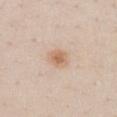Impression:
The lesion was photographed on a routine skin check and not biopsied; there is no pathology result.
Background:
This is a white-light tile. A female subject aged 23–27. A region of skin cropped from a whole-body photographic capture, roughly 15 mm wide. The lesion is on the chest. Automated image analysis of the tile measured a lesion color around L≈65 a*≈18 b*≈32 in CIELAB, a lesion–skin lightness drop of about 9, and a normalized lesion–skin contrast near 7. The analysis additionally found a border-irregularity rating of about 1.5/10, a color-variation rating of about 2.5/10, and peripheral color asymmetry of about 0.5. Measured at roughly 3 mm in maximum diameter.